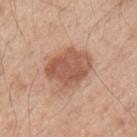Part of a total-body skin-imaging series; this lesion was reviewed on a skin check and was not flagged for biopsy.
Captured under white-light illumination.
The lesion is on the left upper arm.
The subject is a male aged 48–52.
The recorded lesion diameter is about 5.5 mm.
A region of skin cropped from a whole-body photographic capture, roughly 15 mm wide.